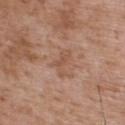Assessment: The lesion was tiled from a total-body skin photograph and was not biopsied. Background: A 15 mm close-up extracted from a 3D total-body photography capture. The subject is a male aged around 50. The lesion's longest dimension is about 3 mm. Imaged with white-light lighting. From the back.